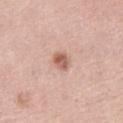biopsy status: catalogued during a skin exam; not biopsied
image: total-body-photography crop, ~15 mm field of view
automated metrics: a lesion color around L≈59 a*≈22 b*≈28 in CIELAB and a lesion-to-skin contrast of about 8 (normalized; higher = more distinct); a within-lesion color-variation index near 4/10 and peripheral color asymmetry of about 1.5; an automated nevus-likeness rating near 80 out of 100 and a detector confidence of about 100 out of 100 that the crop contains a lesion
lesion size: about 2.5 mm
tile lighting: white-light illumination
subject: female, approximately 65 years of age
site: the left thigh